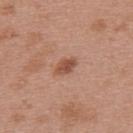Q: Was a biopsy performed?
A: no biopsy performed (imaged during a skin exam)
Q: What is the anatomic site?
A: the upper back
Q: What are the patient's age and sex?
A: female, approximately 40 years of age
Q: Lesion size?
A: ≈3 mm
Q: What is the imaging modality?
A: ~15 mm crop, total-body skin-cancer survey
Q: Automated lesion metrics?
A: a classifier nevus-likeness of about 80/100 and lesion-presence confidence of about 100/100
Q: Illumination type?
A: white-light